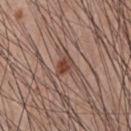biopsy_status: not biopsied; imaged during a skin examination
lighting: white-light
patient:
  sex: male
  age_approx: 60
image:
  source: total-body photography crop
  field_of_view_mm: 15
site: front of the torso
automated_metrics:
  cielab_L: 45
  cielab_a: 20
  cielab_b: 26
  vs_skin_darker_L: 10.0
  vs_skin_contrast_norm: 8.0
  nevus_likeness_0_100: 95